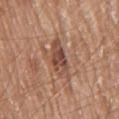This lesion was catalogued during total-body skin photography and was not selected for biopsy. The subject is a male aged approximately 80. The lesion-visualizer software estimated a lesion color around L≈48 a*≈22 b*≈28 in CIELAB and roughly 11 lightness units darker than nearby skin. The analysis additionally found lesion-presence confidence of about 100/100. Captured under white-light illumination. A 15 mm close-up tile from a total-body photography series done for melanoma screening. Measured at roughly 5.5 mm in maximum diameter. Located on the chest.